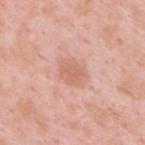Case summary:
• notes · total-body-photography surveillance lesion; no biopsy
• patient · male, aged 33–37
• body site · the upper back
• image source · ~15 mm tile from a whole-body skin photo